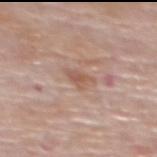Located on the upper back.
This is a white-light tile.
Approximately 3 mm at its widest.
A region of skin cropped from a whole-body photographic capture, roughly 15 mm wide.
A female patient about 65 years old.